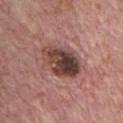{"biopsy_status": "not biopsied; imaged during a skin examination", "site": "chest", "patient": {"sex": "male", "age_approx": 70}, "image": {"source": "total-body photography crop", "field_of_view_mm": 15}, "lighting": "white-light"}